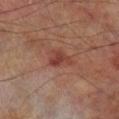Assessment: Recorded during total-body skin imaging; not selected for excision or biopsy. Acquisition and patient details: Cropped from a total-body skin-imaging series; the visible field is about 15 mm. This is a cross-polarized tile. A male patient aged 68 to 72. From the right lower leg. The lesion's longest dimension is about 2.5 mm.